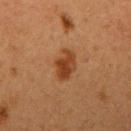Captured during whole-body skin photography for melanoma surveillance; the lesion was not biopsied.